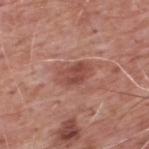Part of a total-body skin-imaging series; this lesion was reviewed on a skin check and was not flagged for biopsy. A male patient aged approximately 60. The lesion is on the upper back. Longest diameter approximately 4 mm. Captured under white-light illumination. Cropped from a whole-body photographic skin survey; the tile spans about 15 mm.